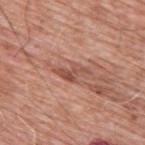No biopsy was performed on this lesion — it was imaged during a full skin examination and was not determined to be concerning.
A male subject approximately 60 years of age.
Imaged with white-light lighting.
The lesion is located on the upper back.
A roughly 15 mm field-of-view crop from a total-body skin photograph.